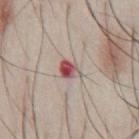{"biopsy_status": "not biopsied; imaged during a skin examination", "lesion_size": {"long_diameter_mm_approx": 3.0}, "site": "chest", "patient": {"sex": "male", "age_approx": 45}, "image": {"source": "total-body photography crop", "field_of_view_mm": 15}, "lighting": "white-light"}